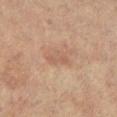biopsy status: catalogued during a skin exam; not biopsied
patient: female, in their 80s
location: the left lower leg
acquisition: ~15 mm tile from a whole-body skin photo